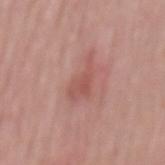The lesion was photographed on a routine skin check and not biopsied; there is no pathology result. The lesion is on the mid back. The recorded lesion diameter is about 4.5 mm. A male patient about 50 years old. Cropped from a total-body skin-imaging series; the visible field is about 15 mm.